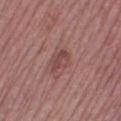Recorded during total-body skin imaging; not selected for excision or biopsy.
A lesion tile, about 15 mm wide, cut from a 3D total-body photograph.
The lesion is located on the right thigh.
Imaged with white-light lighting.
Measured at roughly 3 mm in maximum diameter.
Automated tile analysis of the lesion measured a footprint of about 4.5 mm², a shape eccentricity near 0.65, and a symmetry-axis asymmetry near 0.25. It also reported an average lesion color of about L≈45 a*≈23 b*≈22 (CIELAB), roughly 8 lightness units darker than nearby skin, and a lesion-to-skin contrast of about 6.5 (normalized; higher = more distinct). And it measured a classifier nevus-likeness of about 0/100.
A female subject in their mid- to late 60s.